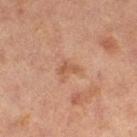<tbp_lesion>
  <biopsy_status>not biopsied; imaged during a skin examination</biopsy_status>
  <automated_metrics>
    <cielab_L>48</cielab_L>
    <cielab_a>20</cielab_a>
    <cielab_b>30</cielab_b>
    <vs_skin_darker_L>7.0</vs_skin_darker_L>
    <vs_skin_contrast_norm>6.0</vs_skin_contrast_norm>
    <border_irregularity_0_10>3.5</border_irregularity_0_10>
    <color_variation_0_10>1.0</color_variation_0_10>
    <peripheral_color_asymmetry>0.5</peripheral_color_asymmetry>
    <nevus_likeness_0_100>0</nevus_likeness_0_100>
    <lesion_detection_confidence_0_100>100</lesion_detection_confidence_0_100>
  </automated_metrics>
  <patient>
    <sex>female</sex>
    <age_approx>60</age_approx>
  </patient>
  <lighting>cross-polarized</lighting>
  <image>
    <source>total-body photography crop</source>
    <field_of_view_mm>15</field_of_view_mm>
  </image>
  <site>right thigh</site>
</tbp_lesion>